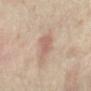follow-up: no biopsy performed (imaged during a skin exam) | patient: female, in their mid-30s | automated metrics: a footprint of about 6.5 mm², a shape eccentricity near 0.8, and two-axis asymmetry of about 0.25; a mean CIELAB color near L≈57 a*≈18 b*≈24, about 8 CIELAB-L* units darker than the surrounding skin, and a lesion-to-skin contrast of about 5.5 (normalized; higher = more distinct); a border-irregularity index near 3/10, a within-lesion color-variation index near 1.5/10, and radial color variation of about 0.5 | anatomic site: the left forearm | diameter: ~3.5 mm (longest diameter) | image: ~15 mm tile from a whole-body skin photo | lighting: cross-polarized.